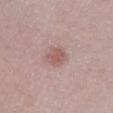Imaged during a routine full-body skin examination; the lesion was not biopsied and no histopathology is available. Automated image analysis of the tile measured a lesion area of about 6 mm², an eccentricity of roughly 0.7, and two-axis asymmetry of about 0.25. The analysis additionally found an average lesion color of about L≈57 a*≈20 b*≈23 (CIELAB), a lesion–skin lightness drop of about 9, and a normalized border contrast of about 6.5. It also reported a within-lesion color-variation index near 2.5/10 and a peripheral color-asymmetry measure near 1. The software also gave an automated nevus-likeness rating near 25 out of 100 and lesion-presence confidence of about 100/100. The subject is a female approximately 25 years of age. The lesion is located on the left lower leg. A 15 mm crop from a total-body photograph taken for skin-cancer surveillance. Imaged with white-light lighting. Approximately 3.5 mm at its widest.